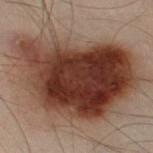Part of a total-body skin-imaging series; this lesion was reviewed on a skin check and was not flagged for biopsy. The tile uses cross-polarized illumination. The recorded lesion diameter is about 13 mm. The patient is a male aged 48–52. The lesion-visualizer software estimated an outline eccentricity of about 0.75 (0 = round, 1 = elongated) and two-axis asymmetry of about 0.3. And it measured a nevus-likeness score of about 100/100 and a detector confidence of about 100 out of 100 that the crop contains a lesion. A close-up tile cropped from a whole-body skin photograph, about 15 mm across. From the left leg.